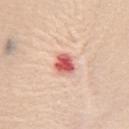The lesion was tiled from a total-body skin photograph and was not biopsied.
Automated tile analysis of the lesion measured a lesion area of about 5.5 mm², an eccentricity of roughly 0.65, and a symmetry-axis asymmetry near 0.15. It also reported a nevus-likeness score of about 0/100.
About 3 mm across.
From the chest.
A female subject roughly 70 years of age.
A 15 mm crop from a total-body photograph taken for skin-cancer surveillance.